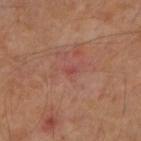The lesion was photographed on a routine skin check and not biopsied; there is no pathology result. A 15 mm close-up extracted from a 3D total-body photography capture. A female subject approximately 60 years of age. This is a cross-polarized tile. On the right upper arm. Automated tile analysis of the lesion measured roughly 6 lightness units darker than nearby skin and a lesion-to-skin contrast of about 5 (normalized; higher = more distinct). The analysis additionally found border irregularity of about 2.5 on a 0–10 scale, a within-lesion color-variation index near 0/10, and a peripheral color-asymmetry measure near 0. And it measured a nevus-likeness score of about 0/100 and a lesion-detection confidence of about 100/100. Longest diameter approximately 1 mm.